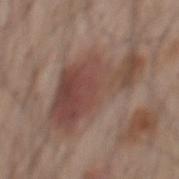Clinical impression:
Recorded during total-body skin imaging; not selected for excision or biopsy.
Context:
A 15 mm close-up extracted from a 3D total-body photography capture. The patient is a male about 60 years old. The total-body-photography lesion software estimated a lesion–skin lightness drop of about 9 and a normalized lesion–skin contrast near 7.5. It also reported border irregularity of about 6.5 on a 0–10 scale and radial color variation of about 1.5. The software also gave lesion-presence confidence of about 100/100. The recorded lesion diameter is about 10 mm. The tile uses white-light illumination. The lesion is on the mid back.